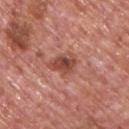The lesion was tiled from a total-body skin photograph and was not biopsied. Approximately 3.5 mm at its widest. The patient is a male aged 73–77. The total-body-photography lesion software estimated a footprint of about 6.5 mm², an eccentricity of roughly 0.75, and a shape-asymmetry score of about 0.35 (0 = symmetric). It also reported border irregularity of about 3.5 on a 0–10 scale and a color-variation rating of about 4.5/10. And it measured a classifier nevus-likeness of about 35/100. Located on the upper back. A 15 mm close-up tile from a total-body photography series done for melanoma screening.